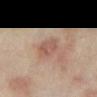The lesion's longest dimension is about 3 mm. Cropped from a whole-body photographic skin survey; the tile spans about 15 mm. Captured under cross-polarized illumination. On the arm. A female patient, in their mid-30s.